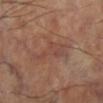Q: Was this lesion biopsied?
A: total-body-photography surveillance lesion; no biopsy
Q: What is the anatomic site?
A: the left lower leg
Q: What lighting was used for the tile?
A: cross-polarized
Q: Automated lesion metrics?
A: an outline eccentricity of about 0.95 (0 = round, 1 = elongated) and a shape-asymmetry score of about 0.55 (0 = symmetric); border irregularity of about 7.5 on a 0–10 scale and a within-lesion color-variation index near 2/10; an automated nevus-likeness rating near 0 out of 100 and a detector confidence of about 80 out of 100 that the crop contains a lesion
Q: How was this image acquired?
A: ~15 mm tile from a whole-body skin photo
Q: Lesion size?
A: ≈5.5 mm